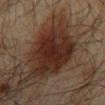workup: catalogued during a skin exam; not biopsied
image-analysis metrics: a footprint of about 27 mm², an outline eccentricity of about 0.75 (0 = round, 1 = elongated), and a symmetry-axis asymmetry near 0.25; a lesion color around L≈25 a*≈18 b*≈23 in CIELAB and a normalized lesion–skin contrast near 12; a border-irregularity index near 3/10, a within-lesion color-variation index near 5/10, and a peripheral color-asymmetry measure near 1.5
image: 15 mm crop, total-body photography
diameter: ≈7.5 mm
location: the mid back
subject: male, about 65 years old
tile lighting: cross-polarized illumination